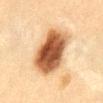Imaged during a routine full-body skin examination; the lesion was not biopsied and no histopathology is available.